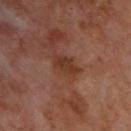notes: total-body-photography surveillance lesion; no biopsy
size: ~4 mm (longest diameter)
image: ~15 mm tile from a whole-body skin photo
illumination: cross-polarized
patient: male, about 70 years old
site: the upper back
automated metrics: an area of roughly 5.5 mm²; roughly 8 lightness units darker than nearby skin and a lesion-to-skin contrast of about 7.5 (normalized; higher = more distinct); a border-irregularity rating of about 2.5/10, internal color variation of about 3 on a 0–10 scale, and a peripheral color-asymmetry measure near 1; a classifier nevus-likeness of about 0/100